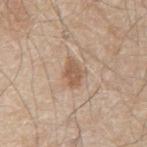{
  "biopsy_status": "not biopsied; imaged during a skin examination",
  "automated_metrics": {
    "vs_skin_darker_L": 10.0,
    "vs_skin_contrast_norm": 7.0
  },
  "patient": {
    "sex": "male",
    "age_approx": 80
  },
  "site": "mid back",
  "image": {
    "source": "total-body photography crop",
    "field_of_view_mm": 15
  },
  "lighting": "white-light"
}